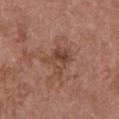biopsy status = imaged on a skin check; not biopsied | subject = female, aged 63 to 67 | imaging modality = total-body-photography crop, ~15 mm field of view | anatomic site = the front of the torso | size = about 3.5 mm | lighting = white-light.